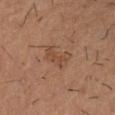  biopsy_status: not biopsied; imaged during a skin examination
  patient:
    sex: male
    age_approx: 55
  image:
    source: total-body photography crop
    field_of_view_mm: 15
  site: right forearm